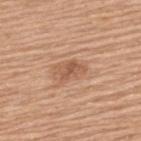Impression:
No biopsy was performed on this lesion — it was imaged during a full skin examination and was not determined to be concerning.